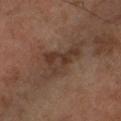Q: Was a biopsy performed?
A: catalogued during a skin exam; not biopsied
Q: What kind of image is this?
A: total-body-photography crop, ~15 mm field of view
Q: Automated lesion metrics?
A: an automated nevus-likeness rating near 0 out of 100
Q: How was the tile lit?
A: cross-polarized
Q: Who is the patient?
A: male, aged approximately 65
Q: What is the anatomic site?
A: the left lower leg